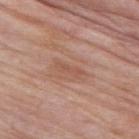• imaging modality: ~15 mm crop, total-body skin-cancer survey
• patient: male, aged around 85 or older
• body site: the right upper arm
• lighting: white-light illumination